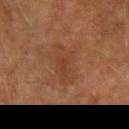- follow-up · no biopsy performed (imaged during a skin exam)
- body site · the left upper arm
- lesion diameter · about 5.5 mm
- lighting · cross-polarized
- patient · male, in their mid- to late 70s
- image source · ~15 mm crop, total-body skin-cancer survey